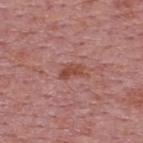Recorded during total-body skin imaging; not selected for excision or biopsy. On the upper back. A 15 mm close-up tile from a total-body photography series done for melanoma screening. The lesion-visualizer software estimated border irregularity of about 4 on a 0–10 scale and peripheral color asymmetry of about 0.5. The analysis additionally found a nevus-likeness score of about 0/100 and a lesion-detection confidence of about 100/100. The subject is a male aged around 75. Approximately 2.5 mm at its widest.